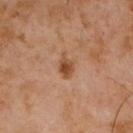The lesion was photographed on a routine skin check and not biopsied; there is no pathology result.
This is a cross-polarized tile.
This image is a 15 mm lesion crop taken from a total-body photograph.
From the chest.
Measured at roughly 3 mm in maximum diameter.
A male subject about 60 years old.